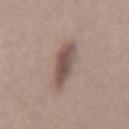Imaged during a routine full-body skin examination; the lesion was not biopsied and no histopathology is available. This is a white-light tile. The lesion is located on the mid back. About 5.5 mm across. A female subject approximately 50 years of age. A 15 mm close-up tile from a total-body photography series done for melanoma screening.